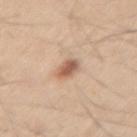From the left thigh.
The subject is a male aged approximately 60.
A 15 mm crop from a total-body photograph taken for skin-cancer surveillance.
About 3 mm across.
Imaged with white-light lighting.
Automated image analysis of the tile measured a lesion area of about 4 mm², an outline eccentricity of about 0.8 (0 = round, 1 = elongated), and a shape-asymmetry score of about 0.15 (0 = symmetric). The analysis additionally found a nevus-likeness score of about 90/100 and a lesion-detection confidence of about 100/100.